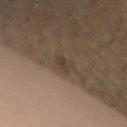Impression:
Recorded during total-body skin imaging; not selected for excision or biopsy.
Context:
This image is a 15 mm lesion crop taken from a total-body photograph. A female subject, aged around 45. The lesion is located on the front of the torso.